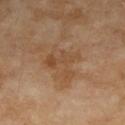Context:
Automated image analysis of the tile measured a nevus-likeness score of about 0/100 and a detector confidence of about 100 out of 100 that the crop contains a lesion. Measured at roughly 4.5 mm in maximum diameter. On the arm. A female patient, aged approximately 70. Cropped from a whole-body photographic skin survey; the tile spans about 15 mm.